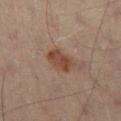Imaged during a routine full-body skin examination; the lesion was not biopsied and no histopathology is available. From the leg. A male patient, in their 60s. Captured under cross-polarized illumination. A region of skin cropped from a whole-body photographic capture, roughly 15 mm wide. Approximately 3 mm at its widest.